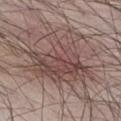{
  "biopsy_status": "not biopsied; imaged during a skin examination",
  "image": {
    "source": "total-body photography crop",
    "field_of_view_mm": 15
  },
  "lesion_size": {
    "long_diameter_mm_approx": 8.5
  },
  "site": "right thigh",
  "patient": {
    "sex": "male",
    "age_approx": 40
  },
  "lighting": "white-light",
  "automated_metrics": {
    "area_mm2_approx": 34.0,
    "shape_asymmetry": 0.5,
    "cielab_L": 47,
    "cielab_a": 17,
    "cielab_b": 20,
    "vs_skin_darker_L": 9.0,
    "vs_skin_contrast_norm": 7.0,
    "lesion_detection_confidence_0_100": 100
  }
}